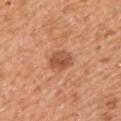workup — total-body-photography surveillance lesion; no biopsy